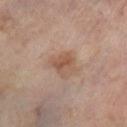Findings:
- notes: imaged on a skin check; not biopsied
- anatomic site: the left lower leg
- size: ≈3 mm
- subject: male, aged around 70
- imaging modality: total-body-photography crop, ~15 mm field of view
- tile lighting: cross-polarized illumination